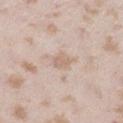<record>
  <biopsy_status>not biopsied; imaged during a skin examination</biopsy_status>
  <image>
    <source>total-body photography crop</source>
    <field_of_view_mm>15</field_of_view_mm>
  </image>
  <patient>
    <sex>female</sex>
    <age_approx>25</age_approx>
  </patient>
  <lighting>white-light</lighting>
  <site>right lower leg</site>
  <lesion_size>
    <long_diameter_mm_approx>2.5</long_diameter_mm_approx>
  </lesion_size>
</record>